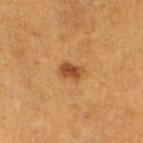biopsy status — catalogued during a skin exam; not biopsied
size — about 3 mm
site — the left lower leg
subject — female, aged 38–42
automated metrics — an area of roughly 4 mm² and a shape eccentricity near 0.75; a lesion color around L≈39 a*≈21 b*≈34 in CIELAB, about 10 CIELAB-L* units darker than the surrounding skin, and a normalized lesion–skin contrast near 8.5; a border-irregularity rating of about 2/10
lighting — cross-polarized illumination
image — ~15 mm crop, total-body skin-cancer survey